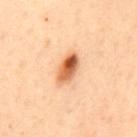Impression:
This lesion was catalogued during total-body skin photography and was not selected for biopsy.
Image and clinical context:
The lesion is located on the mid back. This image is a 15 mm lesion crop taken from a total-body photograph. A male patient, in their mid- to late 40s. The lesion's longest dimension is about 4 mm.